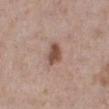notes — catalogued during a skin exam; not biopsied | site — the right lower leg | tile lighting — white-light illumination | subject — female, aged around 60 | diameter — about 3 mm | image source — total-body-photography crop, ~15 mm field of view.